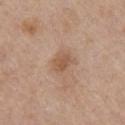This lesion was catalogued during total-body skin photography and was not selected for biopsy.
This image is a 15 mm lesion crop taken from a total-body photograph.
On the front of the torso.
This is a white-light tile.
A male subject, approximately 60 years of age.
The recorded lesion diameter is about 2.5 mm.